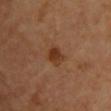Imaged during a routine full-body skin examination; the lesion was not biopsied and no histopathology is available. A subject roughly 70 years of age. A roughly 15 mm field-of-view crop from a total-body skin photograph. On the chest. The lesion's longest dimension is about 2.5 mm.